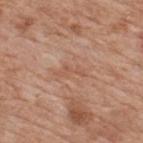Part of a total-body skin-imaging series; this lesion was reviewed on a skin check and was not flagged for biopsy.